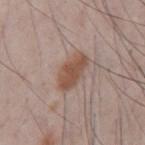workup — imaged on a skin check; not biopsied
anatomic site — the mid back
lesion size — ~4.5 mm (longest diameter)
illumination — white-light
subject — male, about 35 years old
imaging modality — 15 mm crop, total-body photography